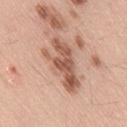The lesion was photographed on a routine skin check and not biopsied; there is no pathology result.
A male patient aged 53 to 57.
On the leg.
Automated tile analysis of the lesion measured a lesion area of about 16 mm² and a shape-asymmetry score of about 0.4 (0 = symmetric). And it measured a mean CIELAB color near L≈57 a*≈22 b*≈29, roughly 14 lightness units darker than nearby skin, and a lesion-to-skin contrast of about 8.5 (normalized; higher = more distinct). The software also gave a border-irregularity index near 6.5/10, internal color variation of about 4.5 on a 0–10 scale, and a peripheral color-asymmetry measure near 1.5. It also reported a nevus-likeness score of about 0/100 and lesion-presence confidence of about 100/100.
Measured at roughly 7 mm in maximum diameter.
Captured under white-light illumination.
A 15 mm crop from a total-body photograph taken for skin-cancer surveillance.